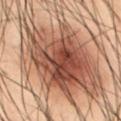notes: no biopsy performed (imaged during a skin exam) | lesion size: ~12.5 mm (longest diameter) | site: the abdomen | automated lesion analysis: roughly 13 lightness units darker than nearby skin and a lesion-to-skin contrast of about 10 (normalized; higher = more distinct) | image source: 15 mm crop, total-body photography | patient: male, roughly 55 years of age.